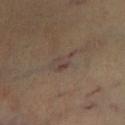Notes:
• lesion size: ≈3.5 mm
• lighting: cross-polarized
• image-analysis metrics: an area of roughly 5 mm², an outline eccentricity of about 0.85 (0 = round, 1 = elongated), and a symmetry-axis asymmetry near 0.35; a color-variation rating of about 4/10; a classifier nevus-likeness of about 0/100 and a detector confidence of about 55 out of 100 that the crop contains a lesion
• anatomic site: the leg
• image source: total-body-photography crop, ~15 mm field of view
• patient: male, roughly 55 years of age